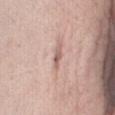Q: Was this lesion biopsied?
A: total-body-photography surveillance lesion; no biopsy
Q: How large is the lesion?
A: ≈2.5 mm
Q: What kind of image is this?
A: ~15 mm tile from a whole-body skin photo
Q: Who is the patient?
A: female, aged approximately 40
Q: How was the tile lit?
A: white-light
Q: Where on the body is the lesion?
A: the abdomen